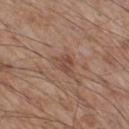Q: Was this lesion biopsied?
A: catalogued during a skin exam; not biopsied
Q: Patient demographics?
A: male, in their mid-50s
Q: How was the tile lit?
A: white-light illumination
Q: What is the anatomic site?
A: the leg
Q: What is the imaging modality?
A: total-body-photography crop, ~15 mm field of view
Q: How large is the lesion?
A: about 3 mm